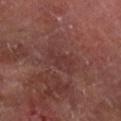Captured during whole-body skin photography for melanoma surveillance; the lesion was not biopsied.
Captured under cross-polarized illumination.
From the right forearm.
A 15 mm close-up tile from a total-body photography series done for melanoma screening.
A male subject roughly 65 years of age.